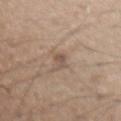| feature | finding |
|---|---|
| lesion diameter | ~3 mm (longest diameter) |
| automated metrics | a border-irregularity rating of about 3/10 and a color-variation rating of about 0/10; a classifier nevus-likeness of about 0/100 and lesion-presence confidence of about 70/100 |
| subject | male, aged approximately 70 |
| tile lighting | white-light |
| imaging modality | ~15 mm crop, total-body skin-cancer survey |
| location | the abdomen |